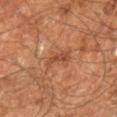Q: Was this lesion biopsied?
A: total-body-photography surveillance lesion; no biopsy
Q: Who is the patient?
A: male, aged 58 to 62
Q: What kind of image is this?
A: ~15 mm tile from a whole-body skin photo
Q: What is the lesion's diameter?
A: about 3 mm
Q: Lesion location?
A: the right leg
Q: Automated lesion metrics?
A: an area of roughly 4 mm², an eccentricity of roughly 0.9, and a shape-asymmetry score of about 0.4 (0 = symmetric)
Q: Illumination type?
A: cross-polarized illumination